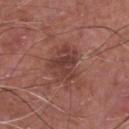Assessment:
Part of a total-body skin-imaging series; this lesion was reviewed on a skin check and was not flagged for biopsy.
Image and clinical context:
This is a white-light tile. A roughly 15 mm field-of-view crop from a total-body skin photograph. The recorded lesion diameter is about 5 mm. The subject is a male in their mid-60s. The lesion is on the chest. An algorithmic analysis of the crop reported a lesion area of about 12 mm², a shape eccentricity near 0.65, and a shape-asymmetry score of about 0.35 (0 = symmetric). The analysis additionally found border irregularity of about 4.5 on a 0–10 scale and a within-lesion color-variation index near 4/10. And it measured an automated nevus-likeness rating near 0 out of 100 and a detector confidence of about 100 out of 100 that the crop contains a lesion.